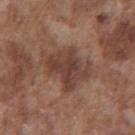{"biopsy_status": "not biopsied; imaged during a skin examination", "image": {"source": "total-body photography crop", "field_of_view_mm": 15}, "patient": {"sex": "male", "age_approx": 75}, "site": "chest", "lesion_size": {"long_diameter_mm_approx": 6.0}, "lighting": "white-light"}